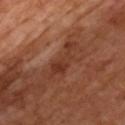Findings:
* notes: total-body-photography surveillance lesion; no biopsy
* acquisition: ~15 mm tile from a whole-body skin photo
* patient: male, roughly 65 years of age
* lesion size: about 5 mm
* tile lighting: cross-polarized
* image-analysis metrics: a footprint of about 6.5 mm², an eccentricity of roughly 0.95, and two-axis asymmetry of about 0.55; an average lesion color of about L≈34 a*≈24 b*≈29 (CIELAB), about 7 CIELAB-L* units darker than the surrounding skin, and a lesion-to-skin contrast of about 6.5 (normalized; higher = more distinct); a nevus-likeness score of about 0/100 and a detector confidence of about 100 out of 100 that the crop contains a lesion